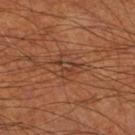workup = imaged on a skin check; not biopsied
lighting = cross-polarized illumination
image source = 15 mm crop, total-body photography
subject = male, in their 70s
size = ≈3 mm
location = the right thigh